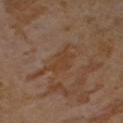Assessment: Recorded during total-body skin imaging; not selected for excision or biopsy. Clinical summary: Captured under cross-polarized illumination. The patient is a female aged approximately 55. A 15 mm close-up tile from a total-body photography series done for melanoma screening. From the chest. The recorded lesion diameter is about 3.5 mm. Automated tile analysis of the lesion measured a lesion area of about 6 mm² and an outline eccentricity of about 0.85 (0 = round, 1 = elongated). And it measured a mean CIELAB color near L≈40 a*≈18 b*≈31, about 5 CIELAB-L* units darker than the surrounding skin, and a normalized border contrast of about 6.5.